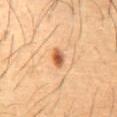The lesion was photographed on a routine skin check and not biopsied; there is no pathology result. Approximately 2.5 mm at its widest. From the front of the torso. Imaged with cross-polarized lighting. The patient is a male aged 58–62. Cropped from a total-body skin-imaging series; the visible field is about 15 mm.